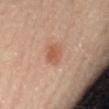Assessment: The lesion was tiled from a total-body skin photograph and was not biopsied. Image and clinical context: Located on the lower back. A 15 mm close-up extracted from a 3D total-body photography capture. The subject is a male about 65 years old.